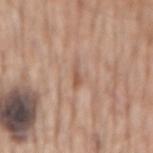The lesion was photographed on a routine skin check and not biopsied; there is no pathology result.
The lesion is on the abdomen.
A male subject about 60 years old.
This is a white-light tile.
Measured at roughly 2.5 mm in maximum diameter.
A 15 mm close-up tile from a total-body photography series done for melanoma screening.
An algorithmic analysis of the crop reported a mean CIELAB color near L≈55 a*≈21 b*≈30 and roughly 9 lightness units darker than nearby skin. The software also gave a classifier nevus-likeness of about 0/100.